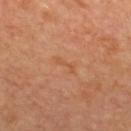| field | value |
|---|---|
| follow-up | catalogued during a skin exam; not biopsied |
| patient | male, in their 60s |
| body site | the back |
| acquisition | 15 mm crop, total-body photography |